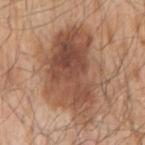workup — catalogued during a skin exam; not biopsied
patient — male, aged 78 to 82
image — ~15 mm crop, total-body skin-cancer survey
anatomic site — the right upper arm
tile lighting — white-light